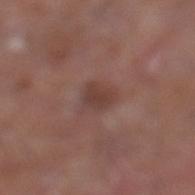{"biopsy_status": "not biopsied; imaged during a skin examination", "lesion_size": {"long_diameter_mm_approx": 3.5}, "automated_metrics": {"nevus_likeness_0_100": 0, "lesion_detection_confidence_0_100": 100}, "site": "left lower leg", "patient": {"sex": "male", "age_approx": 65}, "image": {"source": "total-body photography crop", "field_of_view_mm": 15}}